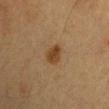Recorded during total-body skin imaging; not selected for excision or biopsy. A 15 mm close-up tile from a total-body photography series done for melanoma screening. The lesion is on the left upper arm. A male patient, roughly 55 years of age. Automated image analysis of the tile measured a nevus-likeness score of about 95/100 and a detector confidence of about 100 out of 100 that the crop contains a lesion. The lesion's longest dimension is about 3 mm. Imaged with cross-polarized lighting.